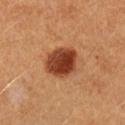Assessment:
Imaged during a routine full-body skin examination; the lesion was not biopsied and no histopathology is available.
Context:
The subject is a female aged 33 to 37. A lesion tile, about 15 mm wide, cut from a 3D total-body photograph. Located on the left forearm. The recorded lesion diameter is about 4.5 mm. This is a cross-polarized tile.